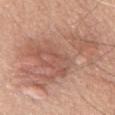Q: Is there a histopathology result?
A: catalogued during a skin exam; not biopsied
Q: What is the anatomic site?
A: the chest
Q: What is the lesion's diameter?
A: ~10.5 mm (longest diameter)
Q: What is the imaging modality?
A: total-body-photography crop, ~15 mm field of view
Q: What lighting was used for the tile?
A: white-light illumination
Q: Who is the patient?
A: male, in their mid- to late 70s